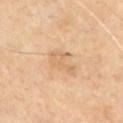Imaged during a routine full-body skin examination; the lesion was not biopsied and no histopathology is available. A male subject, about 70 years old. From the chest. Cropped from a whole-body photographic skin survey; the tile spans about 15 mm.